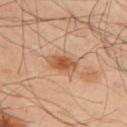Clinical impression:
Part of a total-body skin-imaging series; this lesion was reviewed on a skin check and was not flagged for biopsy.
Background:
A region of skin cropped from a whole-body photographic capture, roughly 15 mm wide. The lesion is located on the back. The patient is a male aged 48–52. Imaged with cross-polarized lighting. About 4 mm across. The lesion-visualizer software estimated an area of roughly 7 mm², a shape eccentricity near 0.85, and a shape-asymmetry score of about 0.2 (0 = symmetric). The analysis additionally found a border-irregularity rating of about 2.5/10, a within-lesion color-variation index near 5.5/10, and a peripheral color-asymmetry measure near 1.5. The analysis additionally found an automated nevus-likeness rating near 90 out of 100.